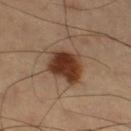This lesion was catalogued during total-body skin photography and was not selected for biopsy.
The patient is a male roughly 60 years of age.
This image is a 15 mm lesion crop taken from a total-body photograph.
Approximately 4.5 mm at its widest.
Located on the right thigh.
The total-body-photography lesion software estimated a lesion area of about 14 mm², a shape eccentricity near 0.4, and two-axis asymmetry of about 0.2. The analysis additionally found a mean CIELAB color near L≈36 a*≈20 b*≈29, about 15 CIELAB-L* units darker than the surrounding skin, and a lesion-to-skin contrast of about 12.5 (normalized; higher = more distinct). The software also gave a border-irregularity index near 2/10, a within-lesion color-variation index near 5/10, and peripheral color asymmetry of about 1.5. The software also gave an automated nevus-likeness rating near 100 out of 100.
Imaged with cross-polarized lighting.